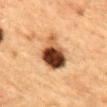Q: Is there a histopathology result?
A: total-body-photography surveillance lesion; no biopsy
Q: Lesion location?
A: the mid back
Q: What kind of image is this?
A: ~15 mm crop, total-body skin-cancer survey
Q: What are the patient's age and sex?
A: male, aged 83 or over
Q: How large is the lesion?
A: about 6 mm
Q: What did automated image analysis measure?
A: a footprint of about 14 mm² and an eccentricity of roughly 0.85